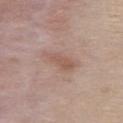follow-up: total-body-photography surveillance lesion; no biopsy
tile lighting: white-light illumination
lesion diameter: ~4 mm (longest diameter)
subject: female, aged approximately 40
acquisition: 15 mm crop, total-body photography
location: the chest